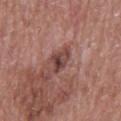No biopsy was performed on this lesion — it was imaged during a full skin examination and was not determined to be concerning. Automated tile analysis of the lesion measured a footprint of about 5 mm² and an outline eccentricity of about 0.75 (0 = round, 1 = elongated). The software also gave border irregularity of about 4 on a 0–10 scale. The lesion is on the head or neck. A male patient, aged 83 or older. A close-up tile cropped from a whole-body skin photograph, about 15 mm across. Imaged with white-light lighting. The lesion's longest dimension is about 3.5 mm.